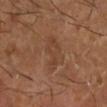Q: Was a biopsy performed?
A: no biopsy performed (imaged during a skin exam)
Q: What is the imaging modality?
A: total-body-photography crop, ~15 mm field of view
Q: Where on the body is the lesion?
A: the right lower leg
Q: Patient demographics?
A: male, in their mid- to late 60s
Q: What did automated image analysis measure?
A: a mean CIELAB color near L≈41 a*≈20 b*≈30, a lesion–skin lightness drop of about 5, and a normalized border contrast of about 4.5; a classifier nevus-likeness of about 0/100 and a lesion-detection confidence of about 100/100